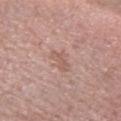workup: imaged on a skin check; not biopsied
automated lesion analysis: a lesion–skin lightness drop of about 7; a border-irregularity rating of about 3.5/10, internal color variation of about 1.5 on a 0–10 scale, and peripheral color asymmetry of about 0.5
anatomic site: the left forearm
subject: female, approximately 60 years of age
imaging modality: 15 mm crop, total-body photography
lesion diameter: ≈3 mm
illumination: white-light illumination